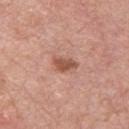The subject is a male aged 53 to 57. Captured under white-light illumination. The lesion is located on the chest. A region of skin cropped from a whole-body photographic capture, roughly 15 mm wide. About 3 mm across.